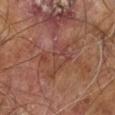notes — imaged on a skin check; not biopsied | patient — male, about 60 years old | acquisition — ~15 mm tile from a whole-body skin photo | image-analysis metrics — an area of roughly 14 mm²; a lesion color around L≈41 a*≈22 b*≈26 in CIELAB and a normalized lesion–skin contrast near 4.5; border irregularity of about 8.5 on a 0–10 scale, a within-lesion color-variation index near 4.5/10, and a peripheral color-asymmetry measure near 1 | illumination — cross-polarized | anatomic site — the leg | lesion size — ≈6.5 mm.